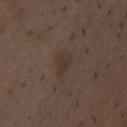{"biopsy_status": "not biopsied; imaged during a skin examination", "lesion_size": {"long_diameter_mm_approx": 2.5}, "patient": {"sex": "male", "age_approx": 50}, "site": "chest", "image": {"source": "total-body photography crop", "field_of_view_mm": 15}, "automated_metrics": {"eccentricity": 0.7, "shape_asymmetry": 0.45, "cielab_L": 32, "cielab_a": 12, "cielab_b": 20, "vs_skin_darker_L": 5.0, "vs_skin_contrast_norm": 5.0, "border_irregularity_0_10": 4.0, "color_variation_0_10": 2.5, "peripheral_color_asymmetry": 1.0}}